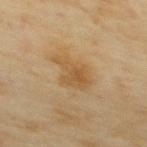Assessment:
The lesion was photographed on a routine skin check and not biopsied; there is no pathology result.
Background:
The lesion is located on the upper back. A female subject, roughly 60 years of age. A 15 mm close-up tile from a total-body photography series done for melanoma screening. This is a cross-polarized tile. Measured at roughly 4.5 mm in maximum diameter.